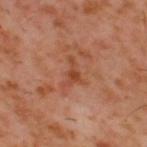Assessment:
No biopsy was performed on this lesion — it was imaged during a full skin examination and was not determined to be concerning.
Background:
Measured at roughly 2.5 mm in maximum diameter. On the upper back. This is a cross-polarized tile. A male patient aged 58 to 62. A 15 mm crop from a total-body photograph taken for skin-cancer surveillance.